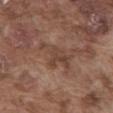biopsy status: catalogued during a skin exam; not biopsied | body site: the abdomen | subject: male, roughly 75 years of age | lesion size: about 5 mm | TBP lesion metrics: a border-irregularity rating of about 7.5/10 and peripheral color asymmetry of about 1; a nevus-likeness score of about 0/100 and a lesion-detection confidence of about 85/100 | tile lighting: white-light illumination | image source: total-body-photography crop, ~15 mm field of view.